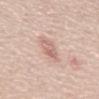The lesion was photographed on a routine skin check and not biopsied; there is no pathology result. The tile uses white-light illumination. The total-body-photography lesion software estimated a within-lesion color-variation index near 4.5/10 and radial color variation of about 2. Cropped from a total-body skin-imaging series; the visible field is about 15 mm. The lesion is on the mid back. A male patient aged 68–72. Approximately 2.5 mm at its widest.